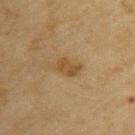Q: Is there a histopathology result?
A: imaged on a skin check; not biopsied
Q: What is the imaging modality?
A: 15 mm crop, total-body photography
Q: Automated lesion metrics?
A: an area of roughly 4.5 mm², a shape eccentricity near 0.75, and a symmetry-axis asymmetry near 0.3; a mean CIELAB color near L≈43 a*≈14 b*≈34, roughly 8 lightness units darker than nearby skin, and a lesion-to-skin contrast of about 7 (normalized; higher = more distinct); a color-variation rating of about 1.5/10 and a peripheral color-asymmetry measure near 0.5; a classifier nevus-likeness of about 20/100 and lesion-presence confidence of about 100/100
Q: Patient demographics?
A: female, aged 53 to 57
Q: Illumination type?
A: cross-polarized
Q: Where on the body is the lesion?
A: the upper back